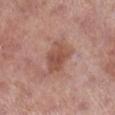<record>
  <biopsy_status>not biopsied; imaged during a skin examination</biopsy_status>
</record>